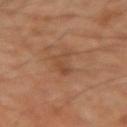Part of a total-body skin-imaging series; this lesion was reviewed on a skin check and was not flagged for biopsy.
From the right upper arm.
Imaged with cross-polarized lighting.
A close-up tile cropped from a whole-body skin photograph, about 15 mm across.
A male subject in their mid-40s.
The lesion's longest dimension is about 4 mm.